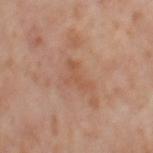workup — total-body-photography surveillance lesion; no biopsy | subject — female, aged around 55 | lesion diameter — about 3.5 mm | imaging modality — ~15 mm crop, total-body skin-cancer survey | lighting — cross-polarized | anatomic site — the right thigh.